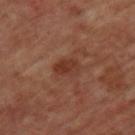notes: no biopsy performed (imaged during a skin exam)
automated lesion analysis: a lesion color around L≈34 a*≈23 b*≈28 in CIELAB, roughly 8 lightness units darker than nearby skin, and a normalized border contrast of about 7; a classifier nevus-likeness of about 10/100 and lesion-presence confidence of about 100/100
subject: female, aged 43 to 47
body site: the back
image: ~15 mm crop, total-body skin-cancer survey
lesion diameter: ~3 mm (longest diameter)
tile lighting: cross-polarized illumination